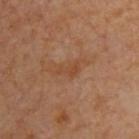The lesion was tiled from a total-body skin photograph and was not biopsied.
The lesion is on the back.
A roughly 15 mm field-of-view crop from a total-body skin photograph.
A patient about 65 years old.
Approximately 2.5 mm at its widest.
The tile uses cross-polarized illumination.